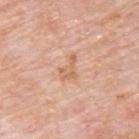| field | value |
|---|---|
| notes | imaged on a skin check; not biopsied |
| image | ~15 mm crop, total-body skin-cancer survey |
| anatomic site | the back |
| patient | male, aged 73–77 |
| lighting | white-light |